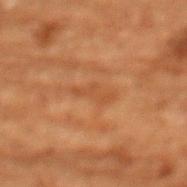<case>
  <biopsy_status>not biopsied; imaged during a skin examination</biopsy_status>
  <patient>
    <sex>female</sex>
    <age_approx>80</age_approx>
  </patient>
  <image>
    <source>total-body photography crop</source>
    <field_of_view_mm>15</field_of_view_mm>
  </image>
  <site>chest</site>
</case>